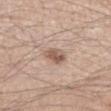The lesion is on the left forearm. The patient is a male aged 33–37. A region of skin cropped from a whole-body photographic capture, roughly 15 mm wide. The lesion-visualizer software estimated a mean CIELAB color near L≈56 a*≈18 b*≈26 and a normalized border contrast of about 8.